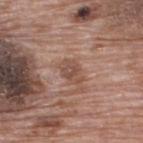| key | value |
|---|---|
| workup | no biopsy performed (imaged during a skin exam) |
| subject | male, roughly 70 years of age |
| anatomic site | the upper back |
| imaging modality | ~15 mm tile from a whole-body skin photo |
| TBP lesion metrics | a lesion area of about 4.5 mm², a shape eccentricity near 0.8, and a symmetry-axis asymmetry near 0.2; a lesion color around L≈49 a*≈20 b*≈26 in CIELAB, about 8 CIELAB-L* units darker than the surrounding skin, and a normalized border contrast of about 6.5; a border-irregularity index near 2.5/10, internal color variation of about 2.5 on a 0–10 scale, and peripheral color asymmetry of about 1; an automated nevus-likeness rating near 0 out of 100 |
| diameter | ≈3 mm |
| lighting | white-light |